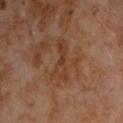| key | value |
|---|---|
| notes | no biopsy performed (imaged during a skin exam) |
| illumination | cross-polarized illumination |
| site | the chest |
| patient | male, aged approximately 60 |
| image | ~15 mm tile from a whole-body skin photo |
| TBP lesion metrics | an area of roughly 6.5 mm², a shape eccentricity near 0.95, and a symmetry-axis asymmetry near 0.5; an average lesion color of about L≈35 a*≈19 b*≈29 (CIELAB) and about 6 CIELAB-L* units darker than the surrounding skin; border irregularity of about 6.5 on a 0–10 scale, a color-variation rating of about 2.5/10, and radial color variation of about 0.5 |